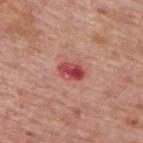Assessment:
Recorded during total-body skin imaging; not selected for excision or biopsy.
Clinical summary:
Measured at roughly 3 mm in maximum diameter. Imaged with white-light lighting. The patient is a male approximately 75 years of age. A region of skin cropped from a whole-body photographic capture, roughly 15 mm wide. On the upper back.